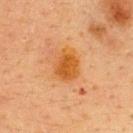follow-up = no biopsy performed (imaged during a skin exam) | acquisition = ~15 mm crop, total-body skin-cancer survey | illumination = cross-polarized | patient = female, about 40 years old | site = the upper back.